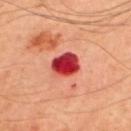The lesion was tiled from a total-body skin photograph and was not biopsied. Longest diameter approximately 3.5 mm. Captured under cross-polarized illumination. On the upper back. A 15 mm close-up extracted from a 3D total-body photography capture. Automated image analysis of the tile measured a mean CIELAB color near L≈45 a*≈47 b*≈32 and about 23 CIELAB-L* units darker than the surrounding skin. The software also gave border irregularity of about 1.5 on a 0–10 scale, internal color variation of about 7.5 on a 0–10 scale, and peripheral color asymmetry of about 2.5. The subject is a male aged approximately 50.